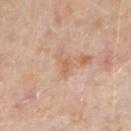Impression: Recorded during total-body skin imaging; not selected for excision or biopsy. Background: A male subject aged 73 to 77. From the right forearm. A lesion tile, about 15 mm wide, cut from a 3D total-body photograph.